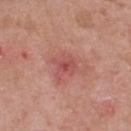Notes:
• biopsy status: total-body-photography surveillance lesion; no biopsy
• subject: male, in their mid- to late 60s
• acquisition: total-body-photography crop, ~15 mm field of view
• location: the upper back